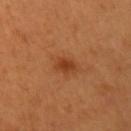follow-up = no biopsy performed (imaged during a skin exam) | image-analysis metrics = border irregularity of about 2.5 on a 0–10 scale, a within-lesion color-variation index near 2.5/10, and a peripheral color-asymmetry measure near 1; a classifier nevus-likeness of about 95/100 and lesion-presence confidence of about 100/100 | site = the left upper arm | size = about 2.5 mm | imaging modality = ~15 mm crop, total-body skin-cancer survey | lighting = cross-polarized | subject = female, aged 43 to 47.